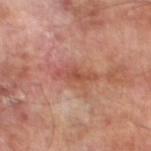Imaged during a routine full-body skin examination; the lesion was not biopsied and no histopathology is available.
A lesion tile, about 15 mm wide, cut from a 3D total-body photograph.
On the left lower leg.
A male patient aged approximately 70.
The lesion-visualizer software estimated a lesion color around L≈50 a*≈26 b*≈29 in CIELAB, a lesion–skin lightness drop of about 8, and a lesion-to-skin contrast of about 6.5 (normalized; higher = more distinct). It also reported border irregularity of about 5 on a 0–10 scale, a color-variation rating of about 3.5/10, and a peripheral color-asymmetry measure near 1.
Captured under cross-polarized illumination.
The lesion's longest dimension is about 4.5 mm.